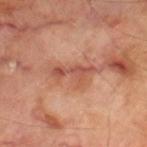Assessment: Imaged during a routine full-body skin examination; the lesion was not biopsied and no histopathology is available. Clinical summary: A male patient, about 70 years old. Imaged with cross-polarized lighting. A 15 mm close-up extracted from a 3D total-body photography capture. The recorded lesion diameter is about 5 mm. The total-body-photography lesion software estimated an area of roughly 6.5 mm², a shape eccentricity near 0.9, and a symmetry-axis asymmetry near 0.5. Located on the left thigh.